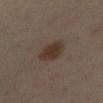{"biopsy_status": "not biopsied; imaged during a skin examination", "lighting": "cross-polarized", "image": {"source": "total-body photography crop", "field_of_view_mm": 15}, "site": "left leg", "automated_metrics": {"area_mm2_approx": 8.0, "eccentricity": 0.25, "shape_asymmetry": 0.15, "vs_skin_darker_L": 9.0, "vs_skin_contrast_norm": 9.0}, "patient": {"sex": "female", "age_approx": 40}}